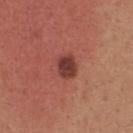Impression:
Recorded during total-body skin imaging; not selected for excision or biopsy.
Clinical summary:
A female patient, aged around 30. A 15 mm crop from a total-body photograph taken for skin-cancer surveillance. Located on the back. An algorithmic analysis of the crop reported a footprint of about 5.5 mm², an outline eccentricity of about 0.65 (0 = round, 1 = elongated), and a shape-asymmetry score of about 0.15 (0 = symmetric). The software also gave a lesion color around L≈39 a*≈27 b*≈26 in CIELAB and roughly 13 lightness units darker than nearby skin. And it measured border irregularity of about 1 on a 0–10 scale, internal color variation of about 3 on a 0–10 scale, and radial color variation of about 1.